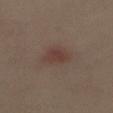Q: Is there a histopathology result?
A: catalogued during a skin exam; not biopsied
Q: What kind of image is this?
A: ~15 mm crop, total-body skin-cancer survey
Q: Patient demographics?
A: female, in their mid- to late 60s
Q: Where on the body is the lesion?
A: the abdomen